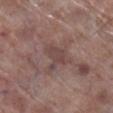Case summary:
• workup — no biopsy performed (imaged during a skin exam)
• imaging modality — total-body-photography crop, ~15 mm field of view
• illumination — white-light
• subject — male, aged around 70
• diameter — ≈3 mm
• automated lesion analysis — a shape eccentricity near 0.6 and a symmetry-axis asymmetry near 0.55; a border-irregularity index near 6.5/10, a within-lesion color-variation index near 1.5/10, and radial color variation of about 0.5
• site — the right lower leg